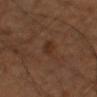The lesion was tiled from a total-body skin photograph and was not biopsied.